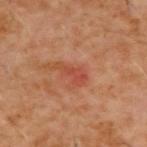<case>
<biopsy_status>not biopsied; imaged during a skin examination</biopsy_status>
<lesion_size>
  <long_diameter_mm_approx>3.5</long_diameter_mm_approx>
</lesion_size>
<site>upper back</site>
<automated_metrics>
  <cielab_L>45</cielab_L>
  <cielab_a>29</cielab_a>
  <cielab_b>32</cielab_b>
  <vs_skin_darker_L>7.0</vs_skin_darker_L>
  <nevus_likeness_0_100>0</nevus_likeness_0_100>
  <lesion_detection_confidence_0_100>100</lesion_detection_confidence_0_100>
</automated_metrics>
<lighting>cross-polarized</lighting>
<patient>
  <sex>male</sex>
  <age_approx>60</age_approx>
</patient>
<image>
  <source>total-body photography crop</source>
  <field_of_view_mm>15</field_of_view_mm>
</image>
</case>